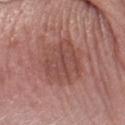Captured during whole-body skin photography for melanoma surveillance; the lesion was not biopsied. A female subject, in their 50s. A roughly 15 mm field-of-view crop from a total-body skin photograph. From the left forearm.